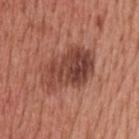Captured during whole-body skin photography for melanoma surveillance; the lesion was not biopsied. On the upper back. The tile uses white-light illumination. A male subject, in their 60s. A 15 mm close-up tile from a total-body photography series done for melanoma screening. Automated tile analysis of the lesion measured a lesion area of about 20 mm² and a symmetry-axis asymmetry near 0.25. It also reported a lesion color around L≈44 a*≈25 b*≈27 in CIELAB, roughly 12 lightness units darker than nearby skin, and a lesion-to-skin contrast of about 9 (normalized; higher = more distinct). And it measured a border-irregularity rating of about 3.5/10, internal color variation of about 7.5 on a 0–10 scale, and a peripheral color-asymmetry measure near 3. About 6.5 mm across.